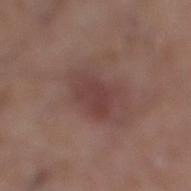Assessment: Imaged during a routine full-body skin examination; the lesion was not biopsied and no histopathology is available. Acquisition and patient details: Automated tile analysis of the lesion measured a lesion color around L≈40 a*≈20 b*≈20 in CIELAB. A male subject about 20 years old. A region of skin cropped from a whole-body photographic capture, roughly 15 mm wide. The lesion is located on the right lower leg. Captured under white-light illumination.